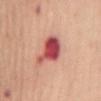<tbp_lesion>
<biopsy_status>not biopsied; imaged during a skin examination</biopsy_status>
<site>mid back</site>
<lighting>white-light</lighting>
<image>
  <source>total-body photography crop</source>
  <field_of_view_mm>15</field_of_view_mm>
</image>
<lesion_size>
  <long_diameter_mm_approx>5.0</long_diameter_mm_approx>
</lesion_size>
<automated_metrics>
  <area_mm2_approx>12.0</area_mm2_approx>
  <shape_asymmetry>0.4</shape_asymmetry>
  <cielab_L>53</cielab_L>
  <cielab_a>35</cielab_a>
  <cielab_b>26</cielab_b>
</automated_metrics>
<patient>
  <sex>female</sex>
  <age_approx>65</age_approx>
</patient>
</tbp_lesion>